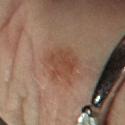The lesion was tiled from a total-body skin photograph and was not biopsied.